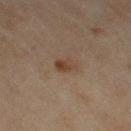Imaged during a routine full-body skin examination; the lesion was not biopsied and no histopathology is available.
The total-body-photography lesion software estimated a symmetry-axis asymmetry near 0.3. The analysis additionally found a lesion–skin lightness drop of about 7 and a lesion-to-skin contrast of about 7 (normalized; higher = more distinct).
A female subject, approximately 60 years of age.
This is a cross-polarized tile.
Longest diameter approximately 2.5 mm.
A close-up tile cropped from a whole-body skin photograph, about 15 mm across.
The lesion is on the left thigh.